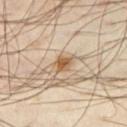The lesion was photographed on a routine skin check and not biopsied; there is no pathology result.
The lesion's longest dimension is about 2.5 mm.
Cropped from a total-body skin-imaging series; the visible field is about 15 mm.
The lesion is on the right thigh.
A male subject, aged 48 to 52.